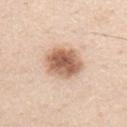Q: Is there a histopathology result?
A: catalogued during a skin exam; not biopsied
Q: Patient demographics?
A: male, aged approximately 45
Q: What is the imaging modality?
A: 15 mm crop, total-body photography
Q: Automated lesion metrics?
A: an automated nevus-likeness rating near 100 out of 100 and lesion-presence confidence of about 100/100
Q: Where on the body is the lesion?
A: the chest
Q: How was the tile lit?
A: white-light illumination
Q: How large is the lesion?
A: ≈4.5 mm